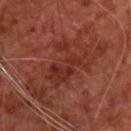| key | value |
|---|---|
| notes | imaged on a skin check; not biopsied |
| image | ~15 mm tile from a whole-body skin photo |
| patient | male, in their mid- to late 60s |
| size | ~6 mm (longest diameter) |
| location | the back |
| lighting | cross-polarized |